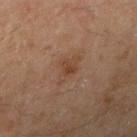Assessment:
Part of a total-body skin-imaging series; this lesion was reviewed on a skin check and was not flagged for biopsy.
Background:
Automated image analysis of the tile measured border irregularity of about 3.5 on a 0–10 scale, a within-lesion color-variation index near 2.5/10, and a peripheral color-asymmetry measure near 0.5. It also reported a nevus-likeness score of about 5/100 and a detector confidence of about 100 out of 100 that the crop contains a lesion. The lesion is located on the right upper arm. A male subject, in their mid-40s. This image is a 15 mm lesion crop taken from a total-body photograph.